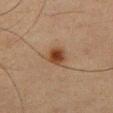Context: Located on the chest. Imaged with cross-polarized lighting. The subject is a male aged around 40. Approximately 3 mm at its widest. This image is a 15 mm lesion crop taken from a total-body photograph.